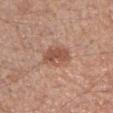Q: Was a biopsy performed?
A: imaged on a skin check; not biopsied